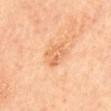Background: The subject is female. Captured under cross-polarized illumination. About 3 mm across. On the mid back. A close-up tile cropped from a whole-body skin photograph, about 15 mm across.